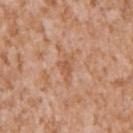biopsy_status: not biopsied; imaged during a skin examination
lesion_size:
  long_diameter_mm_approx: 3.0
site: left upper arm
image:
  source: total-body photography crop
  field_of_view_mm: 15
patient:
  sex: male
  age_approx: 45
lighting: white-light